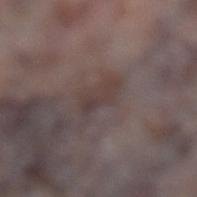The lesion was tiled from a total-body skin photograph and was not biopsied.
The lesion is on the left lower leg.
The lesion's longest dimension is about 4 mm.
This is a white-light tile.
The patient is a female aged 68 to 72.
The lesion-visualizer software estimated a mean CIELAB color near L≈38 a*≈13 b*≈16 and a normalized lesion–skin contrast near 5.5. And it measured border irregularity of about 4 on a 0–10 scale. The software also gave a classifier nevus-likeness of about 0/100.
Cropped from a whole-body photographic skin survey; the tile spans about 15 mm.